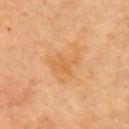Recorded during total-body skin imaging; not selected for excision or biopsy.
Captured under cross-polarized illumination.
Measured at roughly 3 mm in maximum diameter.
Located on the arm.
A region of skin cropped from a whole-body photographic capture, roughly 15 mm wide.
The subject is a female about 70 years old.